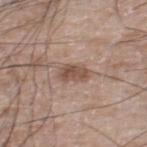Q: Was a biopsy performed?
A: total-body-photography surveillance lesion; no biopsy
Q: What is the anatomic site?
A: the right thigh
Q: How large is the lesion?
A: about 3.5 mm
Q: What did automated image analysis measure?
A: a footprint of about 5.5 mm², a shape eccentricity near 0.75, and a symmetry-axis asymmetry near 0.25; a border-irregularity rating of about 2.5/10 and a within-lesion color-variation index near 3/10; a nevus-likeness score of about 35/100
Q: Patient demographics?
A: male, in their 60s
Q: What kind of image is this?
A: ~15 mm tile from a whole-body skin photo